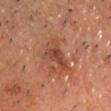Case summary:
- notes · imaged on a skin check; not biopsied
- acquisition · ~15 mm crop, total-body skin-cancer survey
- body site · the head or neck
- patient · male, in their mid-60s
- lesion diameter · about 4 mm
- automated metrics · a lesion area of about 8 mm², an eccentricity of roughly 0.75, and a symmetry-axis asymmetry near 0.5; an average lesion color of about L≈43 a*≈23 b*≈30 (CIELAB), a lesion–skin lightness drop of about 8, and a normalized border contrast of about 6.5; a nevus-likeness score of about 0/100
- lighting · cross-polarized